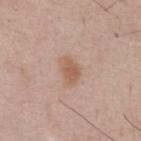  biopsy_status: not biopsied; imaged during a skin examination
  image:
    source: total-body photography crop
    field_of_view_mm: 15
  lesion_size:
    long_diameter_mm_approx: 3.0
  patient:
    sex: male
    age_approx: 55
  lighting: white-light